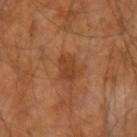| field | value |
|---|---|
| follow-up | total-body-photography surveillance lesion; no biopsy |
| anatomic site | the right upper arm |
| imaging modality | ~15 mm tile from a whole-body skin photo |
| lesion size | ≈3 mm |
| patient | male, approximately 60 years of age |
| image-analysis metrics | a lesion color around L≈40 a*≈23 b*≈34 in CIELAB, about 8 CIELAB-L* units darker than the surrounding skin, and a normalized border contrast of about 6.5 |